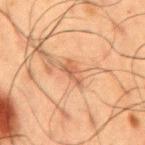<case>
<lesion_size>
  <long_diameter_mm_approx>3.0</long_diameter_mm_approx>
</lesion_size>
<lighting>cross-polarized</lighting>
<site>mid back</site>
<image>
  <source>total-body photography crop</source>
  <field_of_view_mm>15</field_of_view_mm>
</image>
<patient>
  <sex>male</sex>
  <age_approx>60</age_approx>
</patient>
</case>